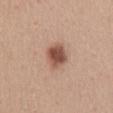Q: Was this lesion biopsied?
A: no biopsy performed (imaged during a skin exam)
Q: Lesion size?
A: ≈3.5 mm
Q: Where on the body is the lesion?
A: the lower back
Q: What is the imaging modality?
A: ~15 mm crop, total-body skin-cancer survey
Q: What are the patient's age and sex?
A: female, approximately 45 years of age